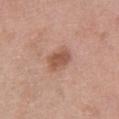Q: Is there a histopathology result?
A: no biopsy performed (imaged during a skin exam)
Q: Where on the body is the lesion?
A: the chest
Q: What kind of image is this?
A: 15 mm crop, total-body photography
Q: Automated lesion metrics?
A: a footprint of about 6.5 mm²; internal color variation of about 2 on a 0–10 scale and a peripheral color-asymmetry measure near 0.5; a classifier nevus-likeness of about 45/100 and a detector confidence of about 100 out of 100 that the crop contains a lesion
Q: Lesion size?
A: ≈3 mm
Q: Illumination type?
A: white-light illumination
Q: Who is the patient?
A: female, approximately 50 years of age